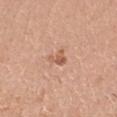No biopsy was performed on this lesion — it was imaged during a full skin examination and was not determined to be concerning.
A 15 mm crop from a total-body photograph taken for skin-cancer surveillance.
A male subject, aged around 50.
An algorithmic analysis of the crop reported a border-irregularity index near 6/10, a color-variation rating of about 0/10, and peripheral color asymmetry of about 0.
The lesion's longest dimension is about 2.5 mm.
The tile uses white-light illumination.
The lesion is on the head or neck.